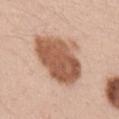The lesion was photographed on a routine skin check and not biopsied; there is no pathology result.
A 15 mm close-up tile from a total-body photography series done for melanoma screening.
Automated image analysis of the tile measured a shape eccentricity near 0.65 and a shape-asymmetry score of about 0.2 (0 = symmetric). The analysis additionally found an average lesion color of about L≈57 a*≈22 b*≈32 (CIELAB), roughly 16 lightness units darker than nearby skin, and a normalized border contrast of about 10.5. And it measured a border-irregularity rating of about 2.5/10 and internal color variation of about 4 on a 0–10 scale.
Approximately 7 mm at its widest.
From the left upper arm.
A female subject aged 38–42.
This is a white-light tile.